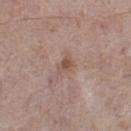workup: total-body-photography surveillance lesion; no biopsy
illumination: white-light
anatomic site: the left thigh
lesion diameter: about 2.5 mm
automated metrics: an average lesion color of about L≈52 a*≈17 b*≈25 (CIELAB), about 8 CIELAB-L* units darker than the surrounding skin, and a normalized lesion–skin contrast near 6.5; a border-irregularity index near 4/10 and a within-lesion color-variation index near 1.5/10; lesion-presence confidence of about 100/100
subject: male, aged 63 to 67
acquisition: ~15 mm tile from a whole-body skin photo